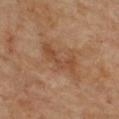An algorithmic analysis of the crop reported a footprint of about 7 mm² and two-axis asymmetry of about 0.65. And it measured a border-irregularity index near 8.5/10 and internal color variation of about 1.5 on a 0–10 scale.
A 15 mm close-up extracted from a 3D total-body photography capture.
A male patient aged 83 or older.
From the upper back.